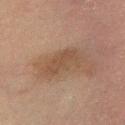Assessment: Part of a total-body skin-imaging series; this lesion was reviewed on a skin check and was not flagged for biopsy. Image and clinical context: Cropped from a whole-body photographic skin survey; the tile spans about 15 mm. About 5 mm across. Imaged with cross-polarized lighting. The patient is a male aged 63–67. Located on the left lower leg. The total-body-photography lesion software estimated a shape eccentricity near 0.9 and a shape-asymmetry score of about 0.25 (0 = symmetric). And it measured a lesion–skin lightness drop of about 7 and a normalized border contrast of about 6. The software also gave internal color variation of about 1.5 on a 0–10 scale and a peripheral color-asymmetry measure near 0.5. The software also gave an automated nevus-likeness rating near 0 out of 100 and a lesion-detection confidence of about 100/100.